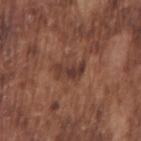No biopsy was performed on this lesion — it was imaged during a full skin examination and was not determined to be concerning.
The tile uses white-light illumination.
The subject is a male in their mid-70s.
A region of skin cropped from a whole-body photographic capture, roughly 15 mm wide.
Automated image analysis of the tile measured a footprint of about 5 mm², a shape eccentricity near 0.9, and a symmetry-axis asymmetry near 0.5. The analysis additionally found an average lesion color of about L≈37 a*≈20 b*≈24 (CIELAB), roughly 9 lightness units darker than nearby skin, and a normalized border contrast of about 8. It also reported a border-irregularity rating of about 5.5/10, a color-variation rating of about 2/10, and peripheral color asymmetry of about 0.5. It also reported a lesion-detection confidence of about 75/100.
Approximately 4 mm at its widest.
Located on the right upper arm.